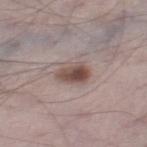Imaged during a routine full-body skin examination; the lesion was not biopsied and no histopathology is available. Automated tile analysis of the lesion measured an average lesion color of about L≈50 a*≈16 b*≈21 (CIELAB) and roughly 12 lightness units darker than nearby skin. And it measured a border-irregularity rating of about 2/10, a within-lesion color-variation index near 7/10, and radial color variation of about 2.5. The analysis additionally found an automated nevus-likeness rating near 95 out of 100. The lesion is on the right thigh. Approximately 3.5 mm at its widest. The tile uses white-light illumination. A close-up tile cropped from a whole-body skin photograph, about 15 mm across. The subject is a male aged around 70.